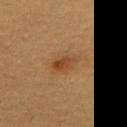workup=catalogued during a skin exam; not biopsied
automated metrics=a lesion area of about 4 mm² and a symmetry-axis asymmetry near 0.2; a mean CIELAB color near L≈38 a*≈20 b*≈33, a lesion–skin lightness drop of about 8, and a normalized border contrast of about 7.5; a border-irregularity rating of about 2/10, internal color variation of about 3 on a 0–10 scale, and a peripheral color-asymmetry measure near 1; an automated nevus-likeness rating near 90 out of 100 and lesion-presence confidence of about 100/100
patient=female, in their 20s
acquisition=~15 mm crop, total-body skin-cancer survey
site=the upper back